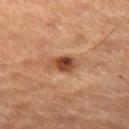The lesion was tiled from a total-body skin photograph and was not biopsied. Captured under cross-polarized illumination. A close-up tile cropped from a whole-body skin photograph, about 15 mm across. On the left thigh. A male subject, roughly 60 years of age.